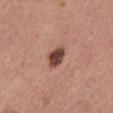Notes:
- biopsy status · total-body-photography surveillance lesion; no biopsy
- acquisition · total-body-photography crop, ~15 mm field of view
- location · the mid back
- patient · male, aged 38–42
- tile lighting · white-light illumination
- diameter · about 2.5 mm